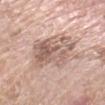Clinical impression: The lesion was tiled from a total-body skin photograph and was not biopsied. Image and clinical context: Approximately 6 mm at its widest. A region of skin cropped from a whole-body photographic capture, roughly 15 mm wide. Captured under white-light illumination. A female patient, aged around 75. The lesion is on the left forearm. An algorithmic analysis of the crop reported an area of roughly 19 mm², an eccentricity of roughly 0.6, and a symmetry-axis asymmetry near 0.3.